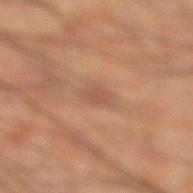Assessment:
Captured during whole-body skin photography for melanoma surveillance; the lesion was not biopsied.
Context:
A close-up tile cropped from a whole-body skin photograph, about 15 mm across. Captured under cross-polarized illumination. Approximately 2.5 mm at its widest. The subject is a male aged approximately 60. On the right lower leg.